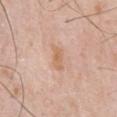Findings:
* TBP lesion metrics — an area of roughly 3.5 mm² and two-axis asymmetry of about 0.3; a lesion–skin lightness drop of about 8 and a normalized lesion–skin contrast near 6.5; a classifier nevus-likeness of about 0/100 and lesion-presence confidence of about 100/100
* tile lighting — white-light
* lesion size — ~3 mm (longest diameter)
* anatomic site — the chest
* acquisition — total-body-photography crop, ~15 mm field of view
* subject — male, approximately 80 years of age